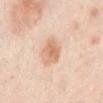Findings:
- workup: catalogued during a skin exam; not biopsied
- body site: the abdomen
- tile lighting: cross-polarized
- lesion size: ~3.5 mm (longest diameter)
- acquisition: ~15 mm crop, total-body skin-cancer survey
- patient: aged around 55